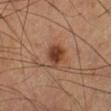Assessment: Imaged during a routine full-body skin examination; the lesion was not biopsied and no histopathology is available. Acquisition and patient details: The patient is a male aged 58–62. A 15 mm close-up extracted from a 3D total-body photography capture. Located on the left lower leg. Approximately 3 mm at its widest. This is a cross-polarized tile.